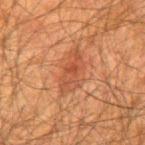notes: catalogued during a skin exam; not biopsied
site: the back
diameter: ~5 mm (longest diameter)
illumination: cross-polarized illumination
patient: male, about 60 years old
automated metrics: an outline eccentricity of about 0.9 (0 = round, 1 = elongated) and a symmetry-axis asymmetry near 0.3; a mean CIELAB color near L≈40 a*≈23 b*≈30 and about 7 CIELAB-L* units darker than the surrounding skin
image: ~15 mm crop, total-body skin-cancer survey